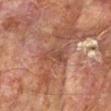Findings:
• follow-up: catalogued during a skin exam; not biopsied
• image-analysis metrics: a lesion–skin lightness drop of about 7 and a normalized border contrast of about 6; a classifier nevus-likeness of about 0/100 and lesion-presence confidence of about 75/100
• patient: male, roughly 75 years of age
• illumination: cross-polarized illumination
• body site: the left forearm
• imaging modality: ~15 mm tile from a whole-body skin photo
• size: about 3.5 mm